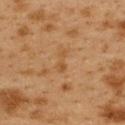Part of a total-body skin-imaging series; this lesion was reviewed on a skin check and was not flagged for biopsy.
The lesion is on the upper back.
The subject is a female aged 38–42.
Cropped from a whole-body photographic skin survey; the tile spans about 15 mm.
The lesion's longest dimension is about 3 mm.
The total-body-photography lesion software estimated a lesion area of about 2.5 mm², a shape eccentricity near 0.95, and two-axis asymmetry of about 0.6.